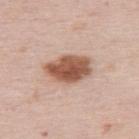This lesion was catalogued during total-body skin photography and was not selected for biopsy.
The subject is a female in their mid-60s.
From the upper back.
The recorded lesion diameter is about 5.5 mm.
Cropped from a whole-body photographic skin survey; the tile spans about 15 mm.
Captured under white-light illumination.